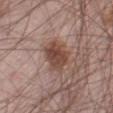| field | value |
|---|---|
| biopsy status | imaged on a skin check; not biopsied |
| automated lesion analysis | a lesion color around L≈46 a*≈19 b*≈25 in CIELAB; a border-irregularity rating of about 2.5/10, internal color variation of about 4 on a 0–10 scale, and peripheral color asymmetry of about 1.5; a nevus-likeness score of about 80/100 and lesion-presence confidence of about 100/100 |
| subject | male, in their mid- to late 60s |
| tile lighting | white-light illumination |
| acquisition | ~15 mm crop, total-body skin-cancer survey |
| anatomic site | the right thigh |